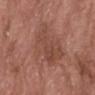Impression:
Part of a total-body skin-imaging series; this lesion was reviewed on a skin check and was not flagged for biopsy.
Background:
A male patient about 65 years old. Automated tile analysis of the lesion measured an average lesion color of about L≈48 a*≈23 b*≈27 (CIELAB). The analysis additionally found a nevus-likeness score of about 0/100 and a lesion-detection confidence of about 100/100. This image is a 15 mm lesion crop taken from a total-body photograph. Imaged with white-light lighting. Measured at roughly 8 mm in maximum diameter. From the head or neck.